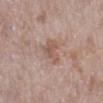biopsy status — total-body-photography surveillance lesion; no biopsy
patient — female, approximately 70 years of age
imaging modality — ~15 mm tile from a whole-body skin photo
location — the left lower leg
lighting — white-light
diameter — about 3.5 mm